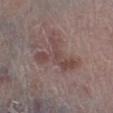No biopsy was performed on this lesion — it was imaged during a full skin examination and was not determined to be concerning.
A male subject aged 78–82.
Measured at roughly 5.5 mm in maximum diameter.
Located on the leg.
A region of skin cropped from a whole-body photographic capture, roughly 15 mm wide.